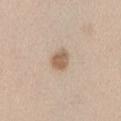The lesion was photographed on a routine skin check and not biopsied; there is no pathology result. This image is a 15 mm lesion crop taken from a total-body photograph. Captured under white-light illumination. A male patient, aged 43 to 47. The lesion is on the left upper arm. The total-body-photography lesion software estimated a border-irregularity index near 1.5/10, a color-variation rating of about 1.5/10, and a peripheral color-asymmetry measure near 0.5.